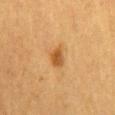Clinical impression:
The lesion was photographed on a routine skin check and not biopsied; there is no pathology result.
Background:
An algorithmic analysis of the crop reported a border-irregularity index near 2.5/10 and peripheral color asymmetry of about 1. About 2.5 mm across. Imaged with cross-polarized lighting. A 15 mm close-up extracted from a 3D total-body photography capture. The patient is a female aged around 55. Located on the mid back.